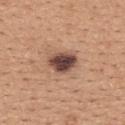{"biopsy_status": "not biopsied; imaged during a skin examination", "automated_metrics": {"border_irregularity_0_10": 1.5, "color_variation_0_10": 5.5}, "lesion_size": {"long_diameter_mm_approx": 3.5}, "patient": {"sex": "female", "age_approx": 30}, "image": {"source": "total-body photography crop", "field_of_view_mm": 15}, "lighting": "white-light", "site": "upper back"}